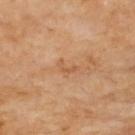Impression:
No biopsy was performed on this lesion — it was imaged during a full skin examination and was not determined to be concerning.
Image and clinical context:
A close-up tile cropped from a whole-body skin photograph, about 15 mm across. This is a cross-polarized tile. Longest diameter approximately 2.5 mm. The lesion is on the upper back.